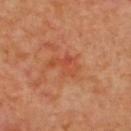Case summary:
• biopsy status — catalogued during a skin exam; not biopsied
• body site — the back
• patient — male, aged approximately 60
• TBP lesion metrics — roughly 6 lightness units darker than nearby skin and a normalized border contrast of about 5; a within-lesion color-variation index near 4/10 and peripheral color asymmetry of about 1.5; an automated nevus-likeness rating near 0 out of 100 and lesion-presence confidence of about 100/100
• image — ~15 mm tile from a whole-body skin photo
• diameter — ≈4 mm